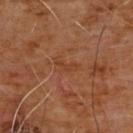<case>
<biopsy_status>not biopsied; imaged during a skin examination</biopsy_status>
<automated_metrics>
  <area_mm2_approx>2.5</area_mm2_approx>
  <eccentricity>0.9</eccentricity>
  <shape_asymmetry>0.55</shape_asymmetry>
  <border_irregularity_0_10>6.0</border_irregularity_0_10>
  <color_variation_0_10>0.0</color_variation_0_10>
  <peripheral_color_asymmetry>0.0</peripheral_color_asymmetry>
</automated_metrics>
<site>chest</site>
<image>
  <source>total-body photography crop</source>
  <field_of_view_mm>15</field_of_view_mm>
</image>
<lesion_size>
  <long_diameter_mm_approx>2.5</long_diameter_mm_approx>
</lesion_size>
<lighting>cross-polarized</lighting>
<patient>
  <sex>male</sex>
  <age_approx>60</age_approx>
</patient>
</case>